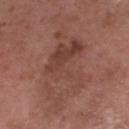{"biopsy_status": "not biopsied; imaged during a skin examination", "image": {"source": "total-body photography crop", "field_of_view_mm": 15}, "automated_metrics": {"cielab_L": 44, "cielab_a": 21, "cielab_b": 25, "nevus_likeness_0_100": 0, "lesion_detection_confidence_0_100": 100}, "site": "head or neck", "lighting": "white-light", "lesion_size": {"long_diameter_mm_approx": 12.5}, "patient": {"sex": "male", "age_approx": 50}}